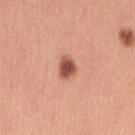Impression:
Part of a total-body skin-imaging series; this lesion was reviewed on a skin check and was not flagged for biopsy.
Context:
The lesion is on the arm. A 15 mm crop from a total-body photograph taken for skin-cancer surveillance. Captured under white-light illumination. Approximately 2.5 mm at its widest. The patient is a female about 30 years old.